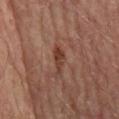notes=imaged on a skin check; not biopsied
image=15 mm crop, total-body photography
lesion size=≈4.5 mm
TBP lesion metrics=a footprint of about 4.5 mm² and an eccentricity of roughly 0.95; an average lesion color of about L≈38 a*≈22 b*≈27 (CIELAB), roughly 8 lightness units darker than nearby skin, and a normalized border contrast of about 7.5
location=the mid back
patient=male, roughly 65 years of age
illumination=cross-polarized